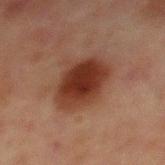{"biopsy_status": "not biopsied; imaged during a skin examination", "image": {"source": "total-body photography crop", "field_of_view_mm": 15}, "site": "chest", "patient": {"sex": "male", "age_approx": 65}}